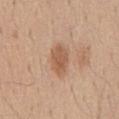{"biopsy_status": "not biopsied; imaged during a skin examination"}